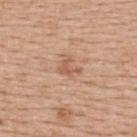notes = catalogued during a skin exam; not biopsied | diameter = ~2.5 mm (longest diameter) | subject = female, approximately 65 years of age | site = the upper back | acquisition = total-body-photography crop, ~15 mm field of view.